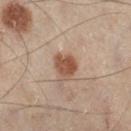Imaged during a routine full-body skin examination; the lesion was not biopsied and no histopathology is available.
The subject is a male in their mid- to late 50s.
A region of skin cropped from a whole-body photographic capture, roughly 15 mm wide.
The total-body-photography lesion software estimated a footprint of about 6.5 mm² and an eccentricity of roughly 0.6. And it measured border irregularity of about 2 on a 0–10 scale, a within-lesion color-variation index near 2.5/10, and radial color variation of about 1. And it measured a classifier nevus-likeness of about 95/100 and a detector confidence of about 100 out of 100 that the crop contains a lesion.
The lesion is on the right lower leg.
Captured under cross-polarized illumination.